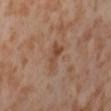follow-up = no biopsy performed (imaged during a skin exam) | image source = ~15 mm crop, total-body skin-cancer survey | lighting = cross-polarized illumination | size = about 3 mm | automated metrics = a mean CIELAB color near L≈47 a*≈20 b*≈31, roughly 8 lightness units darker than nearby skin, and a normalized lesion–skin contrast near 7; a nevus-likeness score of about 0/100 and lesion-presence confidence of about 100/100 | subject = female, about 55 years old | location = the abdomen.